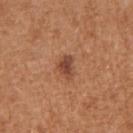{"biopsy_status": "not biopsied; imaged during a skin examination", "patient": {"sex": "female", "age_approx": 40}, "site": "left upper arm", "image": {"source": "total-body photography crop", "field_of_view_mm": 15}}